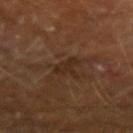Captured during whole-body skin photography for melanoma surveillance; the lesion was not biopsied. A male patient, in their mid- to late 60s. Captured under cross-polarized illumination. From the arm. The total-body-photography lesion software estimated a lesion area of about 4 mm², an eccentricity of roughly 0.85, and a shape-asymmetry score of about 0.5 (0 = symmetric). The software also gave an average lesion color of about L≈25 a*≈16 b*≈25 (CIELAB) and a lesion–skin lightness drop of about 6. The analysis additionally found a color-variation rating of about 1/10. Cropped from a whole-body photographic skin survey; the tile spans about 15 mm.